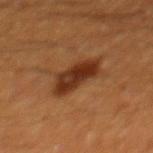Impression:
This lesion was catalogued during total-body skin photography and was not selected for biopsy.
Image and clinical context:
A 15 mm close-up tile from a total-body photography series done for melanoma screening. A male subject aged approximately 60. The lesion is located on the back.